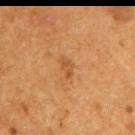This lesion was catalogued during total-body skin photography and was not selected for biopsy.
Located on the right upper arm.
A male patient aged 53 to 57.
A roughly 15 mm field-of-view crop from a total-body skin photograph.
The total-body-photography lesion software estimated a lesion area of about 3 mm², an outline eccentricity of about 0.85 (0 = round, 1 = elongated), and two-axis asymmetry of about 0.4. The analysis additionally found a lesion color around L≈49 a*≈24 b*≈39 in CIELAB, about 7 CIELAB-L* units darker than the surrounding skin, and a normalized border contrast of about 5.5. It also reported a within-lesion color-variation index near 1.5/10. It also reported a classifier nevus-likeness of about 5/100 and a lesion-detection confidence of about 100/100.
The recorded lesion diameter is about 2.5 mm.